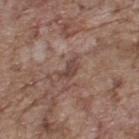{
  "biopsy_status": "not biopsied; imaged during a skin examination",
  "lighting": "white-light",
  "patient": {
    "sex": "male",
    "age_approx": 70
  },
  "site": "upper back",
  "image": {
    "source": "total-body photography crop",
    "field_of_view_mm": 15
  }
}